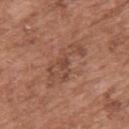follow-up: catalogued during a skin exam; not biopsied
subject: male, aged approximately 75
acquisition: ~15 mm crop, total-body skin-cancer survey
lighting: white-light illumination
anatomic site: the upper back
diameter: ~6.5 mm (longest diameter)